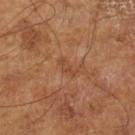Imaged during a routine full-body skin examination; the lesion was not biopsied and no histopathology is available.
Captured under cross-polarized illumination.
A lesion tile, about 15 mm wide, cut from a 3D total-body photograph.
The recorded lesion diameter is about 2.5 mm.
The patient is a male aged 63–67.